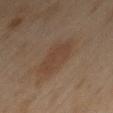workup=imaged on a skin check; not biopsied | subject=female, roughly 60 years of age | location=the right thigh | illumination=cross-polarized illumination | diameter=about 6 mm | image source=~15 mm tile from a whole-body skin photo.